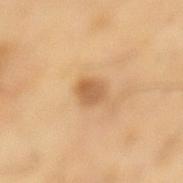| key | value |
|---|---|
| automated metrics | a lesion area of about 5 mm² and a symmetry-axis asymmetry near 0.25; a mean CIELAB color near L≈61 a*≈20 b*≈40, a lesion–skin lightness drop of about 11, and a normalized border contrast of about 7; an automated nevus-likeness rating near 60 out of 100 and lesion-presence confidence of about 100/100 |
| patient | female, in their 70s |
| image source | total-body-photography crop, ~15 mm field of view |
| site | the left lower leg |
| size | about 3 mm |